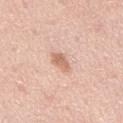The lesion was photographed on a routine skin check and not biopsied; there is no pathology result. The total-body-photography lesion software estimated an average lesion color of about L≈66 a*≈21 b*≈31 (CIELAB) and a lesion-to-skin contrast of about 7.5 (normalized; higher = more distinct). The software also gave a nevus-likeness score of about 75/100 and lesion-presence confidence of about 100/100. A close-up tile cropped from a whole-body skin photograph, about 15 mm across. The patient is a male in their mid- to late 40s. Measured at roughly 2.5 mm in maximum diameter. Captured under white-light illumination. From the back.